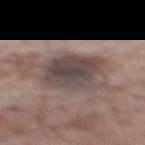Impression: This lesion was catalogued during total-body skin photography and was not selected for biopsy. Background: Located on the back. A male patient, aged approximately 70. Captured under white-light illumination. Longest diameter approximately 6.5 mm. A lesion tile, about 15 mm wide, cut from a 3D total-body photograph.